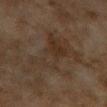tile lighting=cross-polarized illumination
body site=the right upper arm
diameter=~9.5 mm (longest diameter)
acquisition=15 mm crop, total-body photography
subject=female, in their 60s
image-analysis metrics=a border-irregularity rating of about 9/10, a within-lesion color-variation index near 4.5/10, and peripheral color asymmetry of about 1.5; a nevus-likeness score of about 0/100 and a lesion-detection confidence of about 60/100